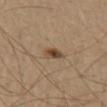| key | value |
|---|---|
| biopsy status | total-body-photography surveillance lesion; no biopsy |